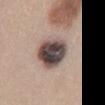The lesion was photographed on a routine skin check and not biopsied; there is no pathology result.